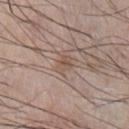Assessment: Recorded during total-body skin imaging; not selected for excision or biopsy. Background: This is a white-light tile. A 15 mm crop from a total-body photograph taken for skin-cancer surveillance. The lesion is on the chest. The subject is a male in their mid-70s. Approximately 2.5 mm at its widest.